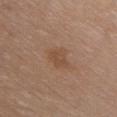notes: imaged on a skin check; not biopsied
lesion size: ~3 mm (longest diameter)
tile lighting: white-light
patient: female, roughly 65 years of age
acquisition: total-body-photography crop, ~15 mm field of view
automated lesion analysis: an area of roughly 4.5 mm² and two-axis asymmetry of about 0.25; border irregularity of about 2.5 on a 0–10 scale and a within-lesion color-variation index near 2/10
anatomic site: the chest